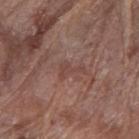follow-up — imaged on a skin check; not biopsied | subject — female, about 75 years old | body site — the arm | image source — total-body-photography crop, ~15 mm field of view | illumination — white-light | diameter — ~3 mm (longest diameter).